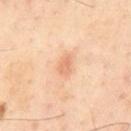follow-up: imaged on a skin check; not biopsied
patient: male, roughly 55 years of age
tile lighting: cross-polarized illumination
lesion diameter: about 2.5 mm
site: the mid back
image: total-body-photography crop, ~15 mm field of view